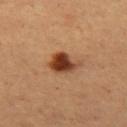Clinical impression: No biopsy was performed on this lesion — it was imaged during a full skin examination and was not determined to be concerning.